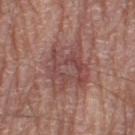This lesion was catalogued during total-body skin photography and was not selected for biopsy.
A male patient aged 78–82.
Located on the leg.
A roughly 15 mm field-of-view crop from a total-body skin photograph.